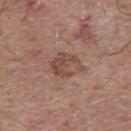| key | value |
|---|---|
| workup | no biopsy performed (imaged during a skin exam) |
| subject | male, in their mid-50s |
| body site | the mid back |
| acquisition | total-body-photography crop, ~15 mm field of view |